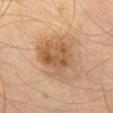Part of a total-body skin-imaging series; this lesion was reviewed on a skin check and was not flagged for biopsy. A male subject, in their mid-60s. A roughly 15 mm field-of-view crop from a total-body skin photograph.